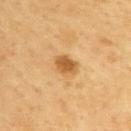From the upper back.
This image is a 15 mm lesion crop taken from a total-body photograph.
A male patient, aged 58 to 62.
The recorded lesion diameter is about 3 mm.